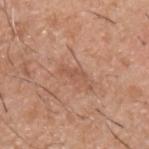- follow-up: imaged on a skin check; not biopsied
- diameter: ≈2.5 mm
- imaging modality: total-body-photography crop, ~15 mm field of view
- patient: male, aged 38 to 42
- body site: the left upper arm
- illumination: white-light
- automated lesion analysis: a footprint of about 2.5 mm², an outline eccentricity of about 0.95 (0 = round, 1 = elongated), and a symmetry-axis asymmetry near 0.45; a mean CIELAB color near L≈54 a*≈23 b*≈31 and a normalized border contrast of about 5; a border-irregularity rating of about 5/10, a within-lesion color-variation index near 0/10, and peripheral color asymmetry of about 0